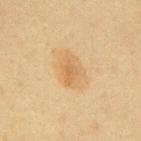biopsy status: catalogued during a skin exam; not biopsied | diameter: ≈5 mm | imaging modality: total-body-photography crop, ~15 mm field of view | lighting: cross-polarized | location: the mid back | TBP lesion metrics: an outline eccentricity of about 0.8 (0 = round, 1 = elongated) and a shape-asymmetry score of about 0.15 (0 = symmetric); a mean CIELAB color near L≈58 a*≈15 b*≈37, a lesion–skin lightness drop of about 7, and a lesion-to-skin contrast of about 5.5 (normalized; higher = more distinct); a within-lesion color-variation index near 3.5/10; a nevus-likeness score of about 85/100 | patient: female, roughly 20 years of age.